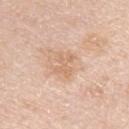Impression:
Captured during whole-body skin photography for melanoma surveillance; the lesion was not biopsied.